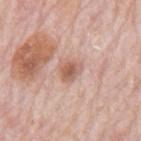Impression: The lesion was tiled from a total-body skin photograph and was not biopsied. Background: A male patient in their 80s. Captured under white-light illumination. This image is a 15 mm lesion crop taken from a total-body photograph. On the mid back.